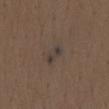workup — no biopsy performed (imaged during a skin exam); lesion diameter — ~3 mm (longest diameter); patient — male, about 75 years old; site — the mid back; tile lighting — white-light illumination; image-analysis metrics — a shape eccentricity near 0.9 and two-axis asymmetry of about 0.3; image — ~15 mm crop, total-body skin-cancer survey.